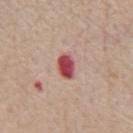lesion size — about 3 mm | acquisition — total-body-photography crop, ~15 mm field of view | subject — male, aged 73 to 77 | body site — the chest.